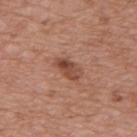The lesion was tiled from a total-body skin photograph and was not biopsied.
A close-up tile cropped from a whole-body skin photograph, about 15 mm across.
A female subject, aged approximately 75.
The lesion is located on the upper back.
Longest diameter approximately 3.5 mm.
The tile uses white-light illumination.